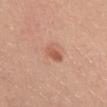Recorded during total-body skin imaging; not selected for excision or biopsy.
On the chest.
Captured under white-light illumination.
A male subject, aged around 30.
Longest diameter approximately 3.5 mm.
A roughly 15 mm field-of-view crop from a total-body skin photograph.
Automated tile analysis of the lesion measured an area of roughly 4 mm², an eccentricity of roughly 0.9, and a shape-asymmetry score of about 0.3 (0 = symmetric). It also reported a nevus-likeness score of about 85/100 and lesion-presence confidence of about 100/100.